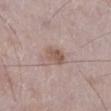{
  "biopsy_status": "not biopsied; imaged during a skin examination",
  "patient": {
    "sex": "male",
    "age_approx": 70
  },
  "lesion_size": {
    "long_diameter_mm_approx": 3.0
  },
  "site": "left lower leg",
  "image": {
    "source": "total-body photography crop",
    "field_of_view_mm": 15
  }
}